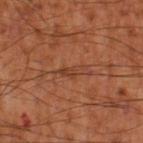No biopsy was performed on this lesion — it was imaged during a full skin examination and was not determined to be concerning.
A lesion tile, about 15 mm wide, cut from a 3D total-body photograph.
Captured under cross-polarized illumination.
Measured at roughly 3 mm in maximum diameter.
The lesion is on the left thigh.
A male patient, aged 53 to 57.